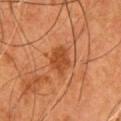- follow-up · catalogued during a skin exam; not biopsied
- patient · male, about 55 years old
- lesion size · about 3.5 mm
- image source · 15 mm crop, total-body photography
- body site · the chest
- automated metrics · a footprint of about 6.5 mm², a shape eccentricity near 0.7, and a shape-asymmetry score of about 0.25 (0 = symmetric); a border-irregularity rating of about 2.5/10 and a within-lesion color-variation index near 2/10; a classifier nevus-likeness of about 70/100 and a lesion-detection confidence of about 100/100